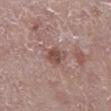Q: Was a biopsy performed?
A: catalogued during a skin exam; not biopsied
Q: What lighting was used for the tile?
A: white-light illumination
Q: Lesion size?
A: about 3 mm
Q: Automated lesion metrics?
A: a lesion area of about 4.5 mm² and a shape-asymmetry score of about 0.25 (0 = symmetric); a normalized lesion–skin contrast near 8; a lesion-detection confidence of about 100/100
Q: Lesion location?
A: the right lower leg
Q: How was this image acquired?
A: total-body-photography crop, ~15 mm field of view
Q: What are the patient's age and sex?
A: female, aged around 65